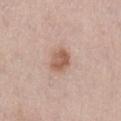subject = male, about 75 years old | lesion size = ≈3 mm | tile lighting = white-light illumination | anatomic site = the left thigh | image source = total-body-photography crop, ~15 mm field of view.